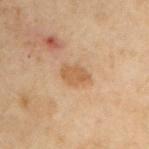Imaged during a routine full-body skin examination; the lesion was not biopsied and no histopathology is available.
On the chest.
A male patient, about 55 years old.
A 15 mm close-up tile from a total-body photography series done for melanoma screening.
The lesion-visualizer software estimated an average lesion color of about L≈47 a*≈16 b*≈31 (CIELAB) and a lesion–skin lightness drop of about 7.